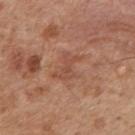No biopsy was performed on this lesion — it was imaged during a full skin examination and was not determined to be concerning. From the mid back. A 15 mm close-up extracted from a 3D total-body photography capture. The lesion's longest dimension is about 3.5 mm. The subject is a male in their mid- to late 40s.